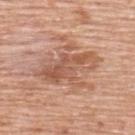Impression: Imaged during a routine full-body skin examination; the lesion was not biopsied and no histopathology is available. Acquisition and patient details: This is a white-light tile. A close-up tile cropped from a whole-body skin photograph, about 15 mm across. Longest diameter approximately 7 mm. The total-body-photography lesion software estimated a lesion area of about 23 mm², an outline eccentricity of about 0.75 (0 = round, 1 = elongated), and a shape-asymmetry score of about 0.4 (0 = symmetric). The analysis additionally found a border-irregularity rating of about 8/10 and internal color variation of about 5.5 on a 0–10 scale. The software also gave a classifier nevus-likeness of about 0/100. A male subject, aged around 60. The lesion is on the back.